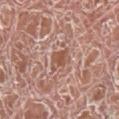Captured during whole-body skin photography for melanoma surveillance; the lesion was not biopsied. This image is a 15 mm lesion crop taken from a total-body photograph. The lesion's longest dimension is about 2.5 mm. The subject is a male aged 73 to 77. Captured under white-light illumination. On the right lower leg.